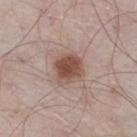Case summary:
• follow-up: catalogued during a skin exam; not biopsied
• subject: male, about 55 years old
• location: the right thigh
• lesion diameter: about 3.5 mm
• automated lesion analysis: an automated nevus-likeness rating near 100 out of 100 and a detector confidence of about 100 out of 100 that the crop contains a lesion
• imaging modality: 15 mm crop, total-body photography